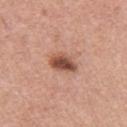This lesion was catalogued during total-body skin photography and was not selected for biopsy. A male patient, in their mid- to late 50s. From the chest. The lesion's longest dimension is about 3.5 mm. A region of skin cropped from a whole-body photographic capture, roughly 15 mm wide.